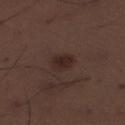Impression: Recorded during total-body skin imaging; not selected for excision or biopsy. Background: From the left lower leg. Longest diameter approximately 3 mm. The patient is a male in their 30s. This image is a 15 mm lesion crop taken from a total-body photograph. The lesion-visualizer software estimated a mean CIELAB color near L≈25 a*≈15 b*≈18, roughly 7 lightness units darker than nearby skin, and a lesion-to-skin contrast of about 8 (normalized; higher = more distinct). The software also gave a nevus-likeness score of about 85/100 and a lesion-detection confidence of about 100/100. The tile uses white-light illumination.